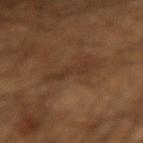Case summary:
- follow-up: total-body-photography surveillance lesion; no biopsy